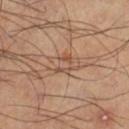The lesion was tiled from a total-body skin photograph and was not biopsied. A male patient, aged 38 to 42. The total-body-photography lesion software estimated an area of roughly 4 mm² and a symmetry-axis asymmetry near 0.45. It also reported a lesion color around L≈51 a*≈19 b*≈29 in CIELAB and a normalized lesion–skin contrast near 5.5. This is a cross-polarized tile. Cropped from a total-body skin-imaging series; the visible field is about 15 mm. From the right lower leg.